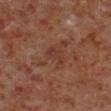Clinical impression: The lesion was tiled from a total-body skin photograph and was not biopsied. Context: Measured at roughly 3 mm in maximum diameter. The lesion-visualizer software estimated internal color variation of about 2 on a 0–10 scale and peripheral color asymmetry of about 0.5. The analysis additionally found a classifier nevus-likeness of about 0/100 and a detector confidence of about 100 out of 100 that the crop contains a lesion. This is a cross-polarized tile. A male subject in their 60s. Located on the right lower leg. A region of skin cropped from a whole-body photographic capture, roughly 15 mm wide.